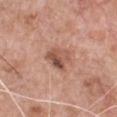Recorded during total-body skin imaging; not selected for excision or biopsy.
Cropped from a whole-body photographic skin survey; the tile spans about 15 mm.
The lesion's longest dimension is about 3 mm.
A male subject, aged 58–62.
This is a white-light tile.
The total-body-photography lesion software estimated a mean CIELAB color near L≈52 a*≈22 b*≈28 and a normalized border contrast of about 8. The software also gave an automated nevus-likeness rating near 10 out of 100.
From the front of the torso.